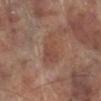A male patient, aged around 70.
Longest diameter approximately 5 mm.
The tile uses white-light illumination.
Located on the left lower leg.
A 15 mm crop from a total-body photograph taken for skin-cancer surveillance.